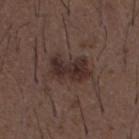The lesion was photographed on a routine skin check and not biopsied; there is no pathology result.
This is a white-light tile.
A male subject, aged 48–52.
The lesion is located on the front of the torso.
A region of skin cropped from a whole-body photographic capture, roughly 15 mm wide.
Automated image analysis of the tile measured an area of roughly 11 mm², an outline eccentricity of about 0.8 (0 = round, 1 = elongated), and a symmetry-axis asymmetry near 0.25.